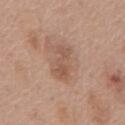– biopsy status · catalogued during a skin exam; not biopsied
– body site · the front of the torso
– image source · ~15 mm crop, total-body skin-cancer survey
– patient · female, in their 60s